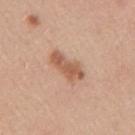Background:
The lesion is on the arm. A female patient in their mid- to late 40s. Automated image analysis of the tile measured a border-irregularity index near 3/10, internal color variation of about 3 on a 0–10 scale, and a peripheral color-asymmetry measure near 1. It also reported a nevus-likeness score of about 75/100 and a detector confidence of about 100 out of 100 that the crop contains a lesion. A region of skin cropped from a whole-body photographic capture, roughly 15 mm wide. Captured under white-light illumination.